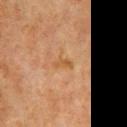illumination: cross-polarized; patient: male, in their 80s; location: the chest; acquisition: ~15 mm tile from a whole-body skin photo; diameter: ≈2.5 mm.